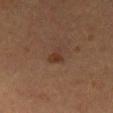workup: total-body-photography surveillance lesion; no biopsy
patient: male, approximately 55 years of age
body site: the mid back
imaging modality: ~15 mm crop, total-body skin-cancer survey